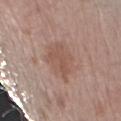The tile uses white-light illumination. An algorithmic analysis of the crop reported an area of roughly 14 mm², a shape eccentricity near 0.65, and a shape-asymmetry score of about 0.25 (0 = symmetric). The analysis additionally found a lesion color around L≈54 a*≈18 b*≈26 in CIELAB, about 7 CIELAB-L* units darker than the surrounding skin, and a lesion-to-skin contrast of about 5.5 (normalized; higher = more distinct). The analysis additionally found a classifier nevus-likeness of about 10/100 and lesion-presence confidence of about 100/100. A 15 mm close-up tile from a total-body photography series done for melanoma screening. A female subject, about 70 years old. About 5 mm across. On the left forearm.